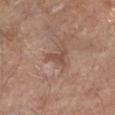Case summary:
– notes — catalogued during a skin exam; not biopsied
– imaging modality — ~15 mm tile from a whole-body skin photo
– anatomic site — the left lower leg
– image-analysis metrics — an area of roughly 3 mm² and an eccentricity of roughly 0.9; a border-irregularity index near 6/10, internal color variation of about 0 on a 0–10 scale, and a peripheral color-asymmetry measure near 0
– illumination — white-light illumination
– patient — male, aged approximately 70